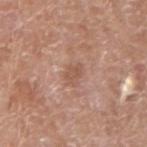Q: How was this image acquired?
A: ~15 mm crop, total-body skin-cancer survey
Q: Lesion location?
A: the right upper arm
Q: Lesion size?
A: about 2.5 mm
Q: What are the patient's age and sex?
A: male, aged around 65